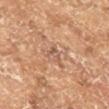biopsy status = total-body-photography surveillance lesion; no biopsy | image source = ~15 mm crop, total-body skin-cancer survey | subject = male, approximately 65 years of age | lesion diameter = about 2.5 mm.